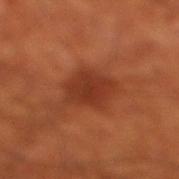The lesion was tiled from a total-body skin photograph and was not biopsied. The tile uses cross-polarized illumination. Cropped from a total-body skin-imaging series; the visible field is about 15 mm. The patient is a male about 70 years old. An algorithmic analysis of the crop reported an outline eccentricity of about 0.7 (0 = round, 1 = elongated). It also reported a border-irregularity rating of about 2/10 and a color-variation rating of about 3.5/10. It also reported a nevus-likeness score of about 85/100 and a lesion-detection confidence of about 100/100. Approximately 5 mm at its widest. The lesion is on the leg.